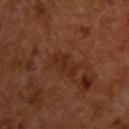image source — 15 mm crop, total-body photography
TBP lesion metrics — an outline eccentricity of about 0.85 (0 = round, 1 = elongated) and two-axis asymmetry of about 0.25; a within-lesion color-variation index near 1.5/10 and peripheral color asymmetry of about 0.5; a classifier nevus-likeness of about 0/100 and lesion-presence confidence of about 100/100
lighting — cross-polarized illumination
subject — male, about 65 years old